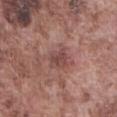biopsy status: total-body-photography surveillance lesion; no biopsy
size: about 2.5 mm
site: the front of the torso
subject: male, aged 73–77
imaging modality: total-body-photography crop, ~15 mm field of view
illumination: white-light illumination